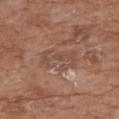Recorded during total-body skin imaging; not selected for excision or biopsy. A female subject aged 73–77. Automated tile analysis of the lesion measured a lesion area of about 9.5 mm² and an outline eccentricity of about 0.9 (0 = round, 1 = elongated). The analysis additionally found a mean CIELAB color near L≈48 a*≈19 b*≈26 and a lesion-to-skin contrast of about 5 (normalized; higher = more distinct). And it measured a border-irregularity index near 8/10, a color-variation rating of about 2.5/10, and peripheral color asymmetry of about 1. This is a white-light tile. Located on the upper back. Longest diameter approximately 5 mm. This image is a 15 mm lesion crop taken from a total-body photograph.